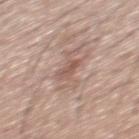<tbp_lesion>
  <biopsy_status>not biopsied; imaged during a skin examination</biopsy_status>
  <lesion_size>
    <long_diameter_mm_approx>5.5</long_diameter_mm_approx>
  </lesion_size>
  <lighting>white-light</lighting>
  <patient>
    <sex>male</sex>
    <age_approx>30</age_approx>
  </patient>
  <image>
    <source>total-body photography crop</source>
    <field_of_view_mm>15</field_of_view_mm>
  </image>
  <automated_metrics>
    <area_mm2_approx>9.0</area_mm2_approx>
    <eccentricity>0.9</eccentricity>
    <shape_asymmetry>0.5</shape_asymmetry>
    <border_irregularity_0_10>9.0</border_irregularity_0_10>
    <peripheral_color_asymmetry>1.0</peripheral_color_asymmetry>
    <nevus_likeness_0_100>0</nevus_likeness_0_100>
  </automated_metrics>
  <site>back</site>
</tbp_lesion>